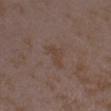Q: Was a biopsy performed?
A: total-body-photography surveillance lesion; no biopsy
Q: Automated lesion metrics?
A: a footprint of about 5 mm², a shape eccentricity near 0.8, and a shape-asymmetry score of about 0.45 (0 = symmetric); a classifier nevus-likeness of about 0/100
Q: What kind of image is this?
A: 15 mm crop, total-body photography
Q: What lighting was used for the tile?
A: white-light illumination
Q: Where on the body is the lesion?
A: the right forearm
Q: Patient demographics?
A: female, aged approximately 35